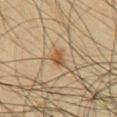Q: Was a biopsy performed?
A: catalogued during a skin exam; not biopsied